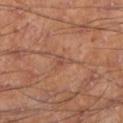| field | value |
|---|---|
| image | 15 mm crop, total-body photography |
| patient | male, about 60 years old |
| lighting | cross-polarized |
| location | the left lower leg |